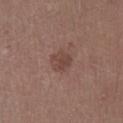Findings:
– workup: imaged on a skin check; not biopsied
– body site: the right lower leg
– image: 15 mm crop, total-body photography
– subject: male, roughly 75 years of age